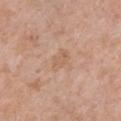Impression:
Recorded during total-body skin imaging; not selected for excision or biopsy.
Background:
From the front of the torso. Imaged with white-light lighting. A region of skin cropped from a whole-body photographic capture, roughly 15 mm wide. A female subject aged around 40. Longest diameter approximately 3 mm.